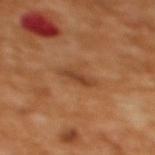Impression: Imaged during a routine full-body skin examination; the lesion was not biopsied and no histopathology is available. Acquisition and patient details: This is a cross-polarized tile. A female patient aged around 55. The lesion is on the upper back. About 3.5 mm across. A 15 mm crop from a total-body photograph taken for skin-cancer surveillance.